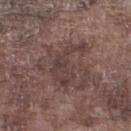Cropped from a total-body skin-imaging series; the visible field is about 15 mm.
The tile uses white-light illumination.
The lesion is on the leg.
A male patient aged around 75.
The total-body-photography lesion software estimated a border-irregularity index near 7.5/10 and a color-variation rating of about 3/10.